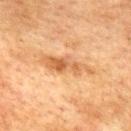Impression: This lesion was catalogued during total-body skin photography and was not selected for biopsy. Clinical summary: A male subject aged 73 to 77. Cropped from a whole-body photographic skin survey; the tile spans about 15 mm. The lesion is on the upper back.